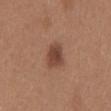No biopsy was performed on this lesion — it was imaged during a full skin examination and was not determined to be concerning. About 3 mm across. The patient is a female aged approximately 30. A region of skin cropped from a whole-body photographic capture, roughly 15 mm wide. Imaged with white-light lighting. Located on the mid back. Automated tile analysis of the lesion measured a footprint of about 7 mm², a shape eccentricity near 0.65, and a shape-asymmetry score of about 0.2 (0 = symmetric). The software also gave a mean CIELAB color near L≈44 a*≈21 b*≈28, about 11 CIELAB-L* units darker than the surrounding skin, and a normalized lesion–skin contrast near 8.5. It also reported border irregularity of about 1.5 on a 0–10 scale and a color-variation rating of about 3/10. The software also gave a lesion-detection confidence of about 100/100.